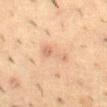No biopsy was performed on this lesion — it was imaged during a full skin examination and was not determined to be concerning. A 15 mm crop from a total-body photograph taken for skin-cancer surveillance. The subject is a male in their mid- to late 50s. The lesion's longest dimension is about 4 mm. The lesion is located on the mid back. The lesion-visualizer software estimated a lesion area of about 5.5 mm² and a shape-asymmetry score of about 0.45 (0 = symmetric). And it measured a border-irregularity rating of about 5/10 and a color-variation rating of about 1.5/10. And it measured a classifier nevus-likeness of about 0/100.